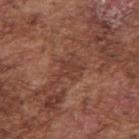follow-up — imaged on a skin check; not biopsied | subject — male, about 75 years old | diameter — ≈3 mm | acquisition — ~15 mm tile from a whole-body skin photo | illumination — white-light illumination | TBP lesion metrics — a nevus-likeness score of about 0/100 and a detector confidence of about 70 out of 100 that the crop contains a lesion | site — the arm.